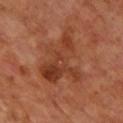| feature | finding |
|---|---|
| lighting | cross-polarized illumination |
| body site | the chest |
| TBP lesion metrics | an automated nevus-likeness rating near 0 out of 100 and a lesion-detection confidence of about 100/100 |
| image source | ~15 mm crop, total-body skin-cancer survey |
| patient | male, aged approximately 65 |